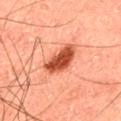follow-up = imaged on a skin check; not biopsied
size = ≈5 mm
automated lesion analysis = a footprint of about 10 mm², a shape eccentricity near 0.85, and two-axis asymmetry of about 0.25; roughly 18 lightness units darker than nearby skin and a normalized border contrast of about 11.5; a border-irregularity rating of about 2.5/10, a within-lesion color-variation index near 4.5/10, and a peripheral color-asymmetry measure near 1.5; a nevus-likeness score of about 100/100 and a detector confidence of about 100 out of 100 that the crop contains a lesion
patient = male, approximately 45 years of age
lighting = cross-polarized
image = ~15 mm tile from a whole-body skin photo
location = the back